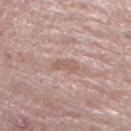patient: male, aged approximately 55 | size: ≈3 mm | site: the left lower leg | tile lighting: white-light illumination | image: 15 mm crop, total-body photography | image-analysis metrics: border irregularity of about 3.5 on a 0–10 scale, internal color variation of about 1 on a 0–10 scale, and radial color variation of about 0; a nevus-likeness score of about 0/100 and lesion-presence confidence of about 70/100.